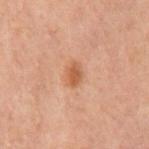Recorded during total-body skin imaging; not selected for excision or biopsy.
A region of skin cropped from a whole-body photographic capture, roughly 15 mm wide.
The subject is a male about 60 years old.
Automated image analysis of the tile measured a lesion–skin lightness drop of about 8 and a normalized border contrast of about 7. The software also gave a nevus-likeness score of about 80/100 and a lesion-detection confidence of about 100/100.
Imaged with cross-polarized lighting.
Located on the chest.
The lesion's longest dimension is about 3 mm.